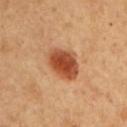Notes:
- lighting · cross-polarized
- automated lesion analysis · a classifier nevus-likeness of about 100/100
- location · the arm
- subject · male, approximately 50 years of age
- imaging modality · ~15 mm tile from a whole-body skin photo
- lesion diameter · ~4 mm (longest diameter)